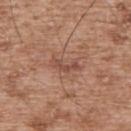Assessment:
No biopsy was performed on this lesion — it was imaged during a full skin examination and was not determined to be concerning.
Image and clinical context:
The tile uses white-light illumination. A 15 mm crop from a total-body photograph taken for skin-cancer surveillance. On the upper back. The subject is a male aged 53–57.